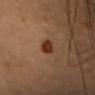Recorded during total-body skin imaging; not selected for excision or biopsy.
Imaged with cross-polarized lighting.
An algorithmic analysis of the crop reported a border-irregularity index near 2/10 and a within-lesion color-variation index near 1.5/10. It also reported a nevus-likeness score of about 100/100.
A close-up tile cropped from a whole-body skin photograph, about 15 mm across.
The lesion is located on the head or neck.
The lesion's longest dimension is about 3 mm.
A female subject, aged 33 to 37.